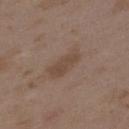{"biopsy_status": "not biopsied; imaged during a skin examination", "site": "upper back", "image": {"source": "total-body photography crop", "field_of_view_mm": 15}, "patient": {"sex": "female", "age_approx": 35}, "lesion_size": {"long_diameter_mm_approx": 4.0}}